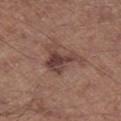notes = imaged on a skin check; not biopsied | subject = male, in their mid- to late 50s | anatomic site = the right lower leg | lesion diameter = ~4.5 mm (longest diameter) | acquisition = total-body-photography crop, ~15 mm field of view | TBP lesion metrics = border irregularity of about 7 on a 0–10 scale, a color-variation rating of about 4.5/10, and a peripheral color-asymmetry measure near 1.5; a nevus-likeness score of about 15/100 and a detector confidence of about 100 out of 100 that the crop contains a lesion | illumination = white-light.